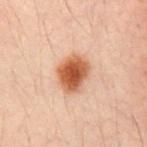Notes:
– follow-up · catalogued during a skin exam; not biopsied
– patient · male, aged approximately 30
– anatomic site · the chest
– acquisition · ~15 mm tile from a whole-body skin photo